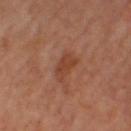Acquisition and patient details: On the right thigh. Captured under cross-polarized illumination. A lesion tile, about 15 mm wide, cut from a 3D total-body photograph. A female subject roughly 65 years of age. Measured at roughly 3.5 mm in maximum diameter.